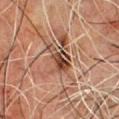Q: Is there a histopathology result?
A: no biopsy performed (imaged during a skin exam)
Q: What did automated image analysis measure?
A: about 11 CIELAB-L* units darker than the surrounding skin
Q: What is the anatomic site?
A: the front of the torso
Q: Who is the patient?
A: male, approximately 50 years of age
Q: What is the imaging modality?
A: 15 mm crop, total-body photography